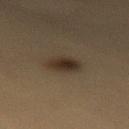The lesion was photographed on a routine skin check and not biopsied; there is no pathology result. A male patient, aged 83 or older. Located on the lower back. This is a cross-polarized tile. A lesion tile, about 15 mm wide, cut from a 3D total-body photograph. Longest diameter approximately 3 mm.